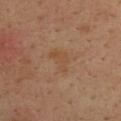{"biopsy_status": "not biopsied; imaged during a skin examination", "patient": {"sex": "male", "age_approx": 35}, "image": {"source": "total-body photography crop", "field_of_view_mm": 15}, "lesion_size": {"long_diameter_mm_approx": 3.0}, "lighting": "cross-polarized", "site": "upper back"}